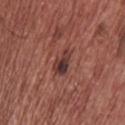follow-up — no biopsy performed (imaged during a skin exam)
image — ~15 mm tile from a whole-body skin photo
size — ≈4 mm
image-analysis metrics — a footprint of about 6 mm² and a shape eccentricity near 0.9; an average lesion color of about L≈37 a*≈22 b*≈22 (CIELAB), about 11 CIELAB-L* units darker than the surrounding skin, and a normalized border contrast of about 9.5; a border-irregularity rating of about 3.5/10, a within-lesion color-variation index near 9.5/10, and radial color variation of about 3.5; a lesion-detection confidence of about 100/100
patient — male, aged 68 to 72
location — the back
tile lighting — white-light